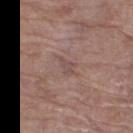biopsy_status: not biopsied; imaged during a skin examination
lesion_size:
  long_diameter_mm_approx: 3.0
image:
  source: total-body photography crop
  field_of_view_mm: 15
patient:
  sex: female
  age_approx: 70
site: right thigh